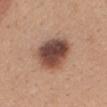No biopsy was performed on this lesion — it was imaged during a full skin examination and was not determined to be concerning. From the mid back. The patient is a female aged 28 to 32. Cropped from a total-body skin-imaging series; the visible field is about 15 mm.